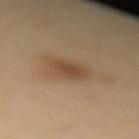{
  "biopsy_status": "not biopsied; imaged during a skin examination",
  "lesion_size": {
    "long_diameter_mm_approx": 3.5
  },
  "image": {
    "source": "total-body photography crop",
    "field_of_view_mm": 15
  },
  "site": "left lower leg",
  "patient": {
    "sex": "female",
    "age_approx": 30
  },
  "lighting": "cross-polarized",
  "automated_metrics": {
    "area_mm2_approx": 7.0,
    "shape_asymmetry": 0.15,
    "cielab_L": 41,
    "cielab_a": 15,
    "cielab_b": 28,
    "vs_skin_contrast_norm": 6.5,
    "nevus_likeness_0_100": 75
  }
}